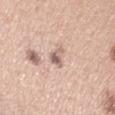Captured during whole-body skin photography for melanoma surveillance; the lesion was not biopsied.
Measured at roughly 3 mm in maximum diameter.
A lesion tile, about 15 mm wide, cut from a 3D total-body photograph.
The lesion-visualizer software estimated an area of roughly 3.5 mm² and an outline eccentricity of about 0.85 (0 = round, 1 = elongated). It also reported a mean CIELAB color near L≈62 a*≈17 b*≈24 and a lesion-to-skin contrast of about 8 (normalized; higher = more distinct).
The patient is a female roughly 30 years of age.
Located on the left upper arm.
The tile uses white-light illumination.